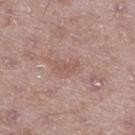notes = imaged on a skin check; not biopsied | diameter = about 2.5 mm | location = the right thigh | patient = male, aged 48–52 | TBP lesion metrics = a shape eccentricity near 0.9 and a symmetry-axis asymmetry near 0.5; about 7 CIELAB-L* units darker than the surrounding skin and a normalized border contrast of about 5; a border-irregularity index near 5.5/10, a within-lesion color-variation index near 0/10, and radial color variation of about 0 | acquisition = ~15 mm tile from a whole-body skin photo | illumination = white-light illumination.